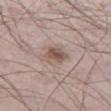anatomic site: the left thigh | size: ~3 mm (longest diameter) | subject: male, aged 68 to 72 | imaging modality: total-body-photography crop, ~15 mm field of view | automated metrics: radial color variation of about 1.5; a classifier nevus-likeness of about 80/100 and lesion-presence confidence of about 100/100.